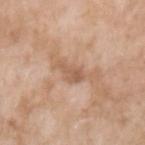illumination: white-light illumination | imaging modality: ~15 mm crop, total-body skin-cancer survey | site: the left upper arm | lesion diameter: ~3.5 mm (longest diameter) | patient: female, roughly 75 years of age | image-analysis metrics: a classifier nevus-likeness of about 0/100.